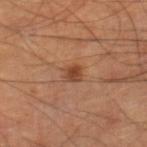notes = no biopsy performed (imaged during a skin exam)
patient = male, about 60 years old
imaging modality = ~15 mm crop, total-body skin-cancer survey
site = the right lower leg
lighting = cross-polarized
lesion size = ~2 mm (longest diameter)